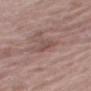Imaged during a routine full-body skin examination; the lesion was not biopsied and no histopathology is available.
This image is a 15 mm lesion crop taken from a total-body photograph.
Approximately 2.5 mm at its widest.
A female patient aged 68 to 72.
The lesion-visualizer software estimated an area of roughly 2.5 mm², an outline eccentricity of about 0.85 (0 = round, 1 = elongated), and a symmetry-axis asymmetry near 0.35. It also reported a border-irregularity rating of about 3.5/10, a within-lesion color-variation index near 1/10, and peripheral color asymmetry of about 0. It also reported an automated nevus-likeness rating near 0 out of 100 and lesion-presence confidence of about 80/100.
The lesion is located on the right thigh.
Captured under white-light illumination.